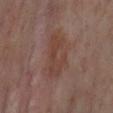notes: catalogued during a skin exam; not biopsied
patient: female, aged 53–57
acquisition: 15 mm crop, total-body photography
site: the right lower leg